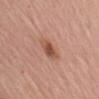This lesion was catalogued during total-body skin photography and was not selected for biopsy. The patient is a male aged around 65. On the right upper arm. The lesion-visualizer software estimated a footprint of about 5 mm², a shape eccentricity near 0.6, and two-axis asymmetry of about 0.25. The software also gave an average lesion color of about L≈52 a*≈24 b*≈30 (CIELAB), roughly 11 lightness units darker than nearby skin, and a normalized border contrast of about 7.5. The software also gave a within-lesion color-variation index near 4/10 and a peripheral color-asymmetry measure near 1.5. Longest diameter approximately 2.5 mm. This is a white-light tile. A region of skin cropped from a whole-body photographic capture, roughly 15 mm wide.